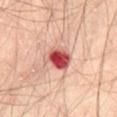This lesion was catalogued during total-body skin photography and was not selected for biopsy. A lesion tile, about 15 mm wide, cut from a 3D total-body photograph. A male subject aged 63 to 67. Imaged with cross-polarized lighting. On the abdomen.